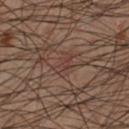Q: Was this lesion biopsied?
A: catalogued during a skin exam; not biopsied
Q: What is the anatomic site?
A: the left lower leg
Q: How was the tile lit?
A: cross-polarized illumination
Q: What kind of image is this?
A: 15 mm crop, total-body photography
Q: Lesion size?
A: about 2.5 mm
Q: Who is the patient?
A: male, roughly 50 years of age
Q: What did automated image analysis measure?
A: about 4 CIELAB-L* units darker than the surrounding skin and a normalized border contrast of about 4.5; a nevus-likeness score of about 0/100 and lesion-presence confidence of about 55/100